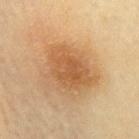Imaged during a routine full-body skin examination; the lesion was not biopsied and no histopathology is available.
A female patient, aged approximately 60.
Cropped from a whole-body photographic skin survey; the tile spans about 15 mm.
Measured at roughly 5.5 mm in maximum diameter.
Imaged with cross-polarized lighting.
On the mid back.